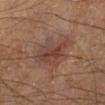  biopsy_status: not biopsied; imaged during a skin examination
  lesion_size:
    long_diameter_mm_approx: 5.5
  patient:
    sex: male
    age_approx: 65
  image:
    source: total-body photography crop
    field_of_view_mm: 15
  automated_metrics:
    area_mm2_approx: 14.0
    eccentricity: 0.8
    border_irregularity_0_10: 4.5
    color_variation_0_10: 4.0
    nevus_likeness_0_100: 35
    lesion_detection_confidence_0_100: 100
  lighting: cross-polarized
  site: left lower leg